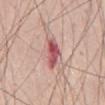Imaged during a routine full-body skin examination; the lesion was not biopsied and no histopathology is available.
A close-up tile cropped from a whole-body skin photograph, about 15 mm across.
A male subject aged 53 to 57.
Longest diameter approximately 3.5 mm.
Located on the abdomen.